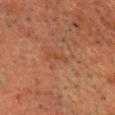Impression: This lesion was catalogued during total-body skin photography and was not selected for biopsy. Background: From the head or neck. A 15 mm close-up tile from a total-body photography series done for melanoma screening. The subject is a male aged 58–62.